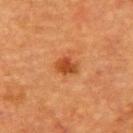This is a cross-polarized tile.
A 15 mm close-up extracted from a 3D total-body photography capture.
Approximately 2.5 mm at its widest.
On the back.
The subject is a female aged approximately 55.